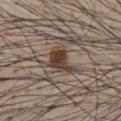Assessment:
Imaged during a routine full-body skin examination; the lesion was not biopsied and no histopathology is available.
Acquisition and patient details:
A male patient, aged 58 to 62. A 15 mm close-up extracted from a 3D total-body photography capture. Approximately 4 mm at its widest. Located on the chest.